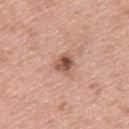Notes:
• site: the upper back
• patient: male, in their 60s
• imaging modality: 15 mm crop, total-body photography
• lesion size: ≈2.5 mm
• lighting: white-light
• TBP lesion metrics: a lesion area of about 5 mm², a shape eccentricity near 0.45, and two-axis asymmetry of about 0.3; an average lesion color of about L≈54 a*≈23 b*≈28 (CIELAB) and a lesion-to-skin contrast of about 8.5 (normalized; higher = more distinct); an automated nevus-likeness rating near 85 out of 100 and a detector confidence of about 100 out of 100 that the crop contains a lesion